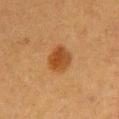Part of a total-body skin-imaging series; this lesion was reviewed on a skin check and was not flagged for biopsy.